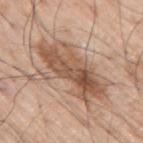Case summary:
- follow-up — no biopsy performed (imaged during a skin exam)
- patient — male, aged approximately 70
- site — the arm
- lighting — white-light
- imaging modality — 15 mm crop, total-body photography